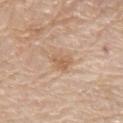Part of a total-body skin-imaging series; this lesion was reviewed on a skin check and was not flagged for biopsy. The lesion is located on the arm. An algorithmic analysis of the crop reported a lesion area of about 4.5 mm² and an outline eccentricity of about 0.7 (0 = round, 1 = elongated). The software also gave a border-irregularity rating of about 3/10, a color-variation rating of about 1.5/10, and peripheral color asymmetry of about 0.5. The analysis additionally found a classifier nevus-likeness of about 20/100. This is a white-light tile. This image is a 15 mm lesion crop taken from a total-body photograph. The patient is a female aged 63–67.